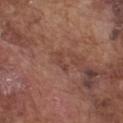Captured during whole-body skin photography for melanoma surveillance; the lesion was not biopsied.
A male patient, about 75 years old.
Cropped from a whole-body photographic skin survey; the tile spans about 15 mm.
From the chest.
Captured under white-light illumination.
The total-body-photography lesion software estimated an area of roughly 2 mm², an eccentricity of roughly 0.95, and two-axis asymmetry of about 0.65. And it measured a lesion color around L≈42 a*≈21 b*≈26 in CIELAB, about 7 CIELAB-L* units darker than the surrounding skin, and a normalized lesion–skin contrast near 5.5. The analysis additionally found a classifier nevus-likeness of about 0/100 and lesion-presence confidence of about 100/100.